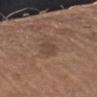Acquisition and patient details:
The recorded lesion diameter is about 3 mm. Located on the arm. Imaged with white-light lighting. A region of skin cropped from a whole-body photographic capture, roughly 15 mm wide. A male patient roughly 65 years of age.